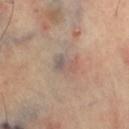No biopsy was performed on this lesion — it was imaged during a full skin examination and was not determined to be concerning. The tile uses cross-polarized illumination. Located on the right thigh. Cropped from a total-body skin-imaging series; the visible field is about 15 mm. Automated image analysis of the tile measured roughly 7 lightness units darker than nearby skin and a normalized lesion–skin contrast near 6. The software also gave a classifier nevus-likeness of about 0/100 and lesion-presence confidence of about 80/100.